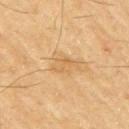Q: What are the patient's age and sex?
A: male, aged 68 to 72
Q: What is the anatomic site?
A: the arm
Q: What is the imaging modality?
A: 15 mm crop, total-body photography